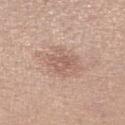Part of a total-body skin-imaging series; this lesion was reviewed on a skin check and was not flagged for biopsy.
Cropped from a whole-body photographic skin survey; the tile spans about 15 mm.
The patient is a female aged 43–47.
Longest diameter approximately 3.5 mm.
The total-body-photography lesion software estimated an eccentricity of roughly 0.45 and a symmetry-axis asymmetry near 0.3. It also reported a mean CIELAB color near L≈59 a*≈19 b*≈25, a lesion–skin lightness drop of about 8, and a lesion-to-skin contrast of about 5.5 (normalized; higher = more distinct). It also reported a border-irregularity index near 4/10, internal color variation of about 3 on a 0–10 scale, and a peripheral color-asymmetry measure near 1. The analysis additionally found a detector confidence of about 100 out of 100 that the crop contains a lesion.
Located on the leg.